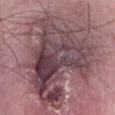Clinical impression:
Imaged during a routine full-body skin examination; the lesion was not biopsied and no histopathology is available.